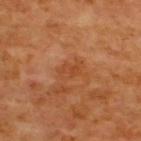{"biopsy_status": "not biopsied; imaged during a skin examination", "lesion_size": {"long_diameter_mm_approx": 3.0}, "lighting": "cross-polarized", "site": "back", "image": {"source": "total-body photography crop", "field_of_view_mm": 15}, "automated_metrics": {"area_mm2_approx": 3.5, "eccentricity": 0.8, "shape_asymmetry": 0.35, "border_irregularity_0_10": 3.5, "color_variation_0_10": 2.5, "peripheral_color_asymmetry": 1.0, "nevus_likeness_0_100": 0, "lesion_detection_confidence_0_100": 100}, "patient": {"sex": "male", "age_approx": 65}}